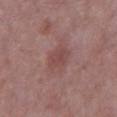Impression: Captured during whole-body skin photography for melanoma surveillance; the lesion was not biopsied. Image and clinical context: A male subject, aged 63–67. Cropped from a whole-body photographic skin survey; the tile spans about 15 mm. The lesion's longest dimension is about 3 mm. The lesion is on the chest.